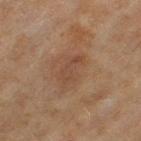biopsy status: no biopsy performed (imaged during a skin exam) | patient: female, aged approximately 60 | anatomic site: the left thigh | image source: ~15 mm crop, total-body skin-cancer survey.